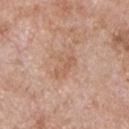notes: imaged on a skin check; not biopsied
image: total-body-photography crop, ~15 mm field of view
site: the chest
illumination: white-light
diameter: about 4 mm
automated lesion analysis: an area of roughly 6.5 mm², an outline eccentricity of about 0.85 (0 = round, 1 = elongated), and a shape-asymmetry score of about 0.3 (0 = symmetric)
patient: male, aged 63 to 67